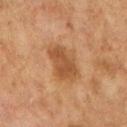Findings:
* workup — total-body-photography surveillance lesion; no biopsy
* automated lesion analysis — an average lesion color of about L≈46 a*≈20 b*≈34 (CIELAB) and a lesion-to-skin contrast of about 7 (normalized; higher = more distinct); a border-irregularity index near 3/10, a color-variation rating of about 3/10, and a peripheral color-asymmetry measure near 1; a classifier nevus-likeness of about 80/100 and lesion-presence confidence of about 100/100
* diameter — ~5.5 mm (longest diameter)
* lighting — cross-polarized
* image — ~15 mm crop, total-body skin-cancer survey
* patient — female, aged approximately 60
* location — the right upper arm